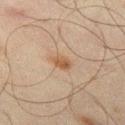The lesion was tiled from a total-body skin photograph and was not biopsied. Automated image analysis of the tile measured a lesion color around L≈45 a*≈15 b*≈29 in CIELAB, a lesion–skin lightness drop of about 8, and a normalized border contrast of about 7.5. It also reported border irregularity of about 2 on a 0–10 scale, a within-lesion color-variation index near 2.5/10, and peripheral color asymmetry of about 1. A male patient, aged 43–47. The recorded lesion diameter is about 3 mm. A close-up tile cropped from a whole-body skin photograph, about 15 mm across. Located on the left thigh.